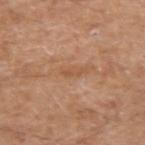follow-up: imaged on a skin check; not biopsied | body site: the left upper arm | acquisition: 15 mm crop, total-body photography | lighting: white-light | subject: male, in their 60s | automated metrics: an outline eccentricity of about 0.95 (0 = round, 1 = elongated); a lesion color around L≈54 a*≈22 b*≈35 in CIELAB and a normalized border contrast of about 5; a nevus-likeness score of about 0/100 and lesion-presence confidence of about 100/100.